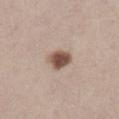Imaged during a routine full-body skin examination; the lesion was not biopsied and no histopathology is available. A female subject aged around 40. On the left lower leg. Cropped from a whole-body photographic skin survey; the tile spans about 15 mm. This is a white-light tile. Longest diameter approximately 3 mm.